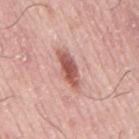biopsy status — total-body-photography surveillance lesion; no biopsy